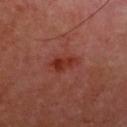Clinical impression: The lesion was tiled from a total-body skin photograph and was not biopsied. Clinical summary: The subject is a male aged 48–52. The tile uses cross-polarized illumination. Automated image analysis of the tile measured an area of roughly 5.5 mm², a shape eccentricity near 0.85, and two-axis asymmetry of about 0.35. A 15 mm close-up extracted from a 3D total-body photography capture. The lesion is on the upper back.